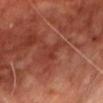<case>
  <biopsy_status>not biopsied; imaged during a skin examination</biopsy_status>
  <site>chest</site>
  <image>
    <source>total-body photography crop</source>
    <field_of_view_mm>15</field_of_view_mm>
  </image>
  <lighting>cross-polarized</lighting>
  <patient>
    <sex>male</sex>
    <age_approx>70</age_approx>
  </patient>
  <lesion_size>
    <long_diameter_mm_approx>3.0</long_diameter_mm_approx>
  </lesion_size>
</case>